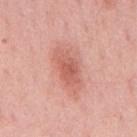Part of a total-body skin-imaging series; this lesion was reviewed on a skin check and was not flagged for biopsy. A region of skin cropped from a whole-body photographic capture, roughly 15 mm wide. Captured under white-light illumination. The lesion is located on the mid back. About 5.5 mm across. The subject is a male approximately 65 years of age.